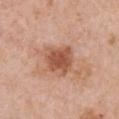Assessment: Part of a total-body skin-imaging series; this lesion was reviewed on a skin check and was not flagged for biopsy. Context: The lesion is located on the front of the torso. Cropped from a total-body skin-imaging series; the visible field is about 15 mm. The tile uses white-light illumination. Approximately 3.5 mm at its widest. The subject is a female roughly 60 years of age.